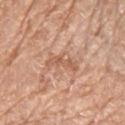Impression: No biopsy was performed on this lesion — it was imaged during a full skin examination and was not determined to be concerning.